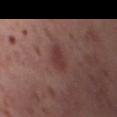follow-up — imaged on a skin check; not biopsied | image source — ~15 mm crop, total-body skin-cancer survey | size — about 3.5 mm | body site — the left thigh | subject — female, aged 53 to 57.